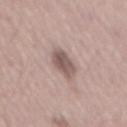Clinical impression:
Captured during whole-body skin photography for melanoma surveillance; the lesion was not biopsied.
Image and clinical context:
A male subject in their mid- to late 50s. Approximately 5 mm at its widest. The tile uses white-light illumination. A roughly 15 mm field-of-view crop from a total-body skin photograph.